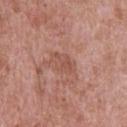biopsy status: no biopsy performed (imaged during a skin exam)
image-analysis metrics: an outline eccentricity of about 0.8 (0 = round, 1 = elongated) and two-axis asymmetry of about 0.15; an automated nevus-likeness rating near 0 out of 100 and a detector confidence of about 100 out of 100 that the crop contains a lesion
subject: male, about 50 years old
lighting: white-light
imaging modality: ~15 mm tile from a whole-body skin photo
body site: the upper back
diameter: about 3.5 mm